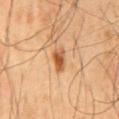Q: Was this lesion biopsied?
A: no biopsy performed (imaged during a skin exam)
Q: What lighting was used for the tile?
A: cross-polarized illumination
Q: What is the imaging modality?
A: ~15 mm crop, total-body skin-cancer survey
Q: What is the anatomic site?
A: the mid back
Q: How large is the lesion?
A: about 3 mm
Q: Patient demographics?
A: male, in their mid-40s